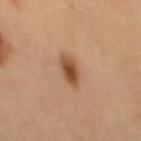Recorded during total-body skin imaging; not selected for excision or biopsy. Approximately 4 mm at its widest. The lesion is located on the mid back. Automated tile analysis of the lesion measured an area of roughly 5.5 mm², an outline eccentricity of about 0.9 (0 = round, 1 = elongated), and a symmetry-axis asymmetry near 0.15. And it measured internal color variation of about 3.5 on a 0–10 scale and radial color variation of about 1. A male subject aged around 60. A 15 mm close-up extracted from a 3D total-body photography capture.